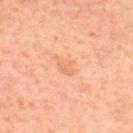| key | value |
|---|---|
| notes | total-body-photography surveillance lesion; no biopsy |
| subject | male, aged 28–32 |
| automated lesion analysis | an area of roughly 4 mm², a shape eccentricity near 0.65, and a symmetry-axis asymmetry near 0.3; border irregularity of about 2.5 on a 0–10 scale |
| lighting | cross-polarized |
| acquisition | ~15 mm crop, total-body skin-cancer survey |
| location | the upper back |
| lesion diameter | ~2.5 mm (longest diameter) |